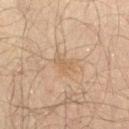The patient is a male about 60 years old.
Imaged with cross-polarized lighting.
The total-body-photography lesion software estimated a mean CIELAB color near L≈45 a*≈13 b*≈27 and roughly 5 lightness units darker than nearby skin. And it measured a border-irregularity index near 4/10, a within-lesion color-variation index near 0/10, and radial color variation of about 0. The software also gave a lesion-detection confidence of about 100/100.
A close-up tile cropped from a whole-body skin photograph, about 15 mm across.
Located on the right thigh.